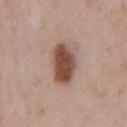No biopsy was performed on this lesion — it was imaged during a full skin examination and was not determined to be concerning. The total-body-photography lesion software estimated border irregularity of about 2 on a 0–10 scale and internal color variation of about 4 on a 0–10 scale. The analysis additionally found a classifier nevus-likeness of about 100/100 and a lesion-detection confidence of about 100/100. A male patient, aged 48–52. Located on the front of the torso. Measured at roughly 5 mm in maximum diameter. Cropped from a whole-body photographic skin survey; the tile spans about 15 mm. Captured under white-light illumination.